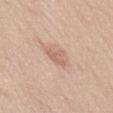| key | value |
|---|---|
| biopsy status | imaged on a skin check; not biopsied |
| image | 15 mm crop, total-body photography |
| lighting | white-light illumination |
| subject | male, about 30 years old |
| site | the abdomen |